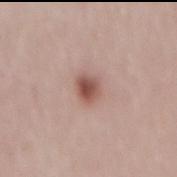Acquisition and patient details: Approximately 2.5 mm at its widest. On the mid back. The subject is a female roughly 30 years of age. Cropped from a whole-body photographic skin survey; the tile spans about 15 mm. Automated tile analysis of the lesion measured a footprint of about 5 mm² and a shape-asymmetry score of about 0.2 (0 = symmetric). It also reported an average lesion color of about L≈52 a*≈22 b*≈24 (CIELAB), about 13 CIELAB-L* units darker than the surrounding skin, and a normalized lesion–skin contrast near 9. The software also gave border irregularity of about 1.5 on a 0–10 scale and a within-lesion color-variation index near 4.5/10. The software also gave a classifier nevus-likeness of about 95/100 and lesion-presence confidence of about 100/100.